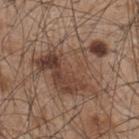tile lighting: white-light illumination
diameter: ~9 mm (longest diameter)
acquisition: ~15 mm tile from a whole-body skin photo
automated metrics: an average lesion color of about L≈44 a*≈17 b*≈26 (CIELAB), roughly 9 lightness units darker than nearby skin, and a normalized border contrast of about 7.5; border irregularity of about 7.5 on a 0–10 scale, internal color variation of about 9 on a 0–10 scale, and peripheral color asymmetry of about 3.5; a classifier nevus-likeness of about 15/100
body site: the upper back
subject: male, aged 43 to 47